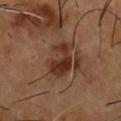Imaged during a routine full-body skin examination; the lesion was not biopsied and no histopathology is available. Located on the front of the torso. A male patient, in their mid-50s. This image is a 15 mm lesion crop taken from a total-body photograph. Imaged with cross-polarized lighting. About 5 mm across.